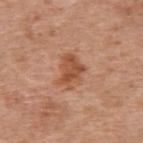No biopsy was performed on this lesion — it was imaged during a full skin examination and was not determined to be concerning. This image is a 15 mm lesion crop taken from a total-body photograph. A male patient approximately 60 years of age. The lesion is located on the upper back.